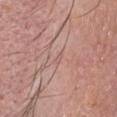biopsy status: no biopsy performed (imaged during a skin exam)
illumination: white-light illumination
diameter: about 1.5 mm
anatomic site: the head or neck
patient: female, in their mid- to late 50s
image source: total-body-photography crop, ~15 mm field of view